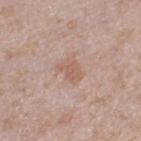Captured during whole-body skin photography for melanoma surveillance; the lesion was not biopsied. Cropped from a whole-body photographic skin survey; the tile spans about 15 mm. Imaged with white-light lighting. The lesion is located on the left thigh. Approximately 2.5 mm at its widest. A female subject, in their 60s.